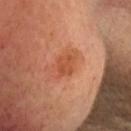notes: catalogued during a skin exam; not biopsied | patient: female, aged 43 to 47 | body site: the head or neck | image source: ~15 mm crop, total-body skin-cancer survey | tile lighting: cross-polarized illumination | lesion size: ≈2.5 mm.